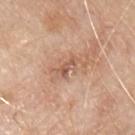Notes:
• notes — total-body-photography surveillance lesion; no biopsy
• automated lesion analysis — an eccentricity of roughly 0.85; roughly 9 lightness units darker than nearby skin and a lesion-to-skin contrast of about 6.5 (normalized; higher = more distinct)
• site — the chest
• subject — male, aged 78–82
• image source — total-body-photography crop, ~15 mm field of view